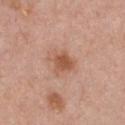workup=imaged on a skin check; not biopsied | diameter=≈3.5 mm | site=the chest | imaging modality=15 mm crop, total-body photography | lighting=white-light illumination | subject=female, in their mid- to late 30s.